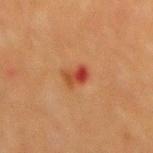Image and clinical context: The tile uses cross-polarized illumination. The lesion-visualizer software estimated an area of roughly 4.5 mm², an eccentricity of roughly 0.8, and two-axis asymmetry of about 0.3. And it measured a mean CIELAB color near L≈38 a*≈27 b*≈32, about 9 CIELAB-L* units darker than the surrounding skin, and a normalized lesion–skin contrast near 8. And it measured a border-irregularity index near 3/10, internal color variation of about 10 on a 0–10 scale, and a peripheral color-asymmetry measure near 5. A male subject, about 50 years old. A 15 mm close-up extracted from a 3D total-body photography capture. The lesion is on the mid back. The lesion's longest dimension is about 3 mm.